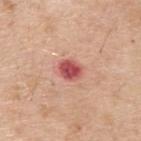biopsy status=no biopsy performed (imaged during a skin exam) | acquisition=total-body-photography crop, ~15 mm field of view | subject=male, in their 70s | image-analysis metrics=a footprint of about 5.5 mm² and a shape eccentricity near 0.55; border irregularity of about 1.5 on a 0–10 scale, a color-variation rating of about 4.5/10, and peripheral color asymmetry of about 2; an automated nevus-likeness rating near 0 out of 100 | site=the upper back | size=~3 mm (longest diameter).